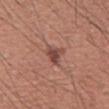- follow-up: no biopsy performed (imaged during a skin exam)
- subject: male, approximately 45 years of age
- image: ~15 mm tile from a whole-body skin photo
- lighting: white-light illumination
- size: ~3 mm (longest diameter)
- location: the mid back